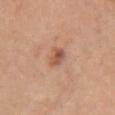The lesion was photographed on a routine skin check and not biopsied; there is no pathology result.